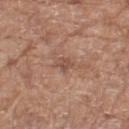{
  "biopsy_status": "not biopsied; imaged during a skin examination"
}